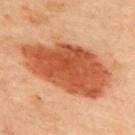A roughly 15 mm field-of-view crop from a total-body skin photograph. The subject is a male aged approximately 45. The lesion is on the upper back.